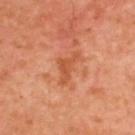Recorded during total-body skin imaging; not selected for excision or biopsy. From the upper back. An algorithmic analysis of the crop reported a footprint of about 5 mm², a shape eccentricity near 0.85, and a shape-asymmetry score of about 0.65 (0 = symmetric). The software also gave about 9 CIELAB-L* units darker than the surrounding skin and a normalized lesion–skin contrast near 6.5. And it measured a border-irregularity index near 7/10, a within-lesion color-variation index near 1.5/10, and peripheral color asymmetry of about 0.5. A lesion tile, about 15 mm wide, cut from a 3D total-body photograph. The subject is a male aged approximately 50. This is a cross-polarized tile.